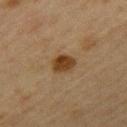{
  "biopsy_status": "not biopsied; imaged during a skin examination",
  "patient": {
    "sex": "female",
    "age_approx": 45
  },
  "image": {
    "source": "total-body photography crop",
    "field_of_view_mm": 15
  },
  "lesion_size": {
    "long_diameter_mm_approx": 3.0
  },
  "site": "upper back",
  "automated_metrics": {
    "eccentricity": 0.7,
    "shape_asymmetry": 0.2,
    "cielab_L": 34,
    "cielab_a": 17,
    "cielab_b": 31,
    "vs_skin_contrast_norm": 10.5,
    "border_irregularity_0_10": 2.0,
    "color_variation_0_10": 2.5,
    "peripheral_color_asymmetry": 0.5
  }
}